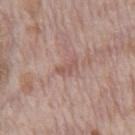<tbp_lesion>
  <biopsy_status>not biopsied; imaged during a skin examination</biopsy_status>
  <lighting>white-light</lighting>
  <image>
    <source>total-body photography crop</source>
    <field_of_view_mm>15</field_of_view_mm>
  </image>
  <patient>
    <sex>male</sex>
    <age_approx>70</age_approx>
  </patient>
  <site>abdomen</site>
</tbp_lesion>